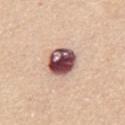Impression:
No biopsy was performed on this lesion — it was imaged during a full skin examination and was not determined to be concerning.
Clinical summary:
On the chest. A female patient roughly 65 years of age. Imaged with white-light lighting. A lesion tile, about 15 mm wide, cut from a 3D total-body photograph. Longest diameter approximately 4 mm.